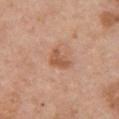notes: no biopsy performed (imaged during a skin exam)
subject: female, roughly 60 years of age
site: the chest
image source: 15 mm crop, total-body photography
lesion diameter: ~3 mm (longest diameter)